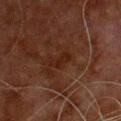Q: Is there a histopathology result?
A: no biopsy performed (imaged during a skin exam)
Q: Lesion size?
A: ≈3 mm
Q: Who is the patient?
A: male, approximately 80 years of age
Q: What is the imaging modality?
A: ~15 mm tile from a whole-body skin photo
Q: How was the tile lit?
A: cross-polarized
Q: What is the anatomic site?
A: the chest
Q: Automated lesion metrics?
A: a footprint of about 3 mm²; a mean CIELAB color near L≈17 a*≈17 b*≈21 and a normalized lesion–skin contrast near 6.5; a border-irregularity index near 4/10 and a color-variation rating of about 0/10; a detector confidence of about 100 out of 100 that the crop contains a lesion